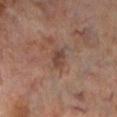Notes:
* biopsy status: total-body-photography surveillance lesion; no biopsy
* lighting: cross-polarized
* acquisition: ~15 mm crop, total-body skin-cancer survey
* patient: male, roughly 70 years of age
* diameter: ≈2.5 mm
* automated metrics: a lesion area of about 3.5 mm² and a shape eccentricity near 0.8; a lesion color around L≈41 a*≈18 b*≈24 in CIELAB and a normalized lesion–skin contrast near 7
* location: the left lower leg